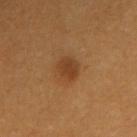Assessment:
Captured during whole-body skin photography for melanoma surveillance; the lesion was not biopsied.
Clinical summary:
Captured under cross-polarized illumination. Located on the left arm. Cropped from a total-body skin-imaging series; the visible field is about 15 mm. The lesion's longest dimension is about 3 mm. A female subject in their 30s.